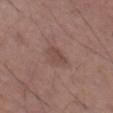{"biopsy_status": "not biopsied; imaged during a skin examination", "patient": {"sex": "male", "age_approx": 70}, "image": {"source": "total-body photography crop", "field_of_view_mm": 15}, "site": "left thigh"}